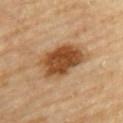Q: Was a biopsy performed?
A: no biopsy performed (imaged during a skin exam)
Q: What are the patient's age and sex?
A: male, aged 83 or over
Q: What is the imaging modality?
A: ~15 mm tile from a whole-body skin photo
Q: What is the anatomic site?
A: the upper back